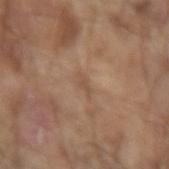The lesion was tiled from a total-body skin photograph and was not biopsied. The total-body-photography lesion software estimated about 6 CIELAB-L* units darker than the surrounding skin and a normalized lesion–skin contrast near 4.5. A male patient aged 78 to 82. The lesion is located on the left forearm. The tile uses white-light illumination. Cropped from a whole-body photographic skin survey; the tile spans about 15 mm. The recorded lesion diameter is about 2.5 mm.